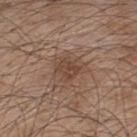{
  "biopsy_status": "not biopsied; imaged during a skin examination",
  "patient": {
    "sex": "male",
    "age_approx": 45
  },
  "image": {
    "source": "total-body photography crop",
    "field_of_view_mm": 15
  },
  "lesion_size": {
    "long_diameter_mm_approx": 3.5
  },
  "site": "upper back"
}